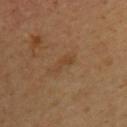Impression:
Captured during whole-body skin photography for melanoma surveillance; the lesion was not biopsied.
Context:
A roughly 15 mm field-of-view crop from a total-body skin photograph. A female patient, in their 40s. The recorded lesion diameter is about 3.5 mm. From the upper back.